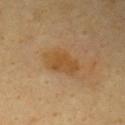Impression:
The lesion was tiled from a total-body skin photograph and was not biopsied.
Clinical summary:
Longest diameter approximately 4.5 mm. Captured under cross-polarized illumination. A 15 mm close-up tile from a total-body photography series done for melanoma screening. The lesion is on the front of the torso. The subject is a male in their mid- to late 60s.